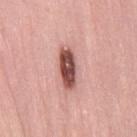Clinical summary: On the right thigh. A female patient, aged 38–42. Captured under white-light illumination. An algorithmic analysis of the crop reported a footprint of about 8.5 mm², an outline eccentricity of about 0.9 (0 = round, 1 = elongated), and a symmetry-axis asymmetry near 0.2. The software also gave a classifier nevus-likeness of about 100/100 and a detector confidence of about 100 out of 100 that the crop contains a lesion. A 15 mm close-up tile from a total-body photography series done for melanoma screening. Longest diameter approximately 5 mm.